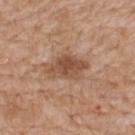Q: Was a biopsy performed?
A: catalogued during a skin exam; not biopsied
Q: How was this image acquired?
A: total-body-photography crop, ~15 mm field of view
Q: Illumination type?
A: white-light illumination
Q: What is the anatomic site?
A: the mid back
Q: Patient demographics?
A: male, in their 60s
Q: What is the lesion's diameter?
A: ~4.5 mm (longest diameter)